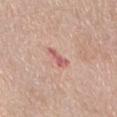Captured during whole-body skin photography for melanoma surveillance; the lesion was not biopsied.
From the front of the torso.
A female patient, aged 73 to 77.
A region of skin cropped from a whole-body photographic capture, roughly 15 mm wide.
The lesion's longest dimension is about 3 mm.
This is a white-light tile.